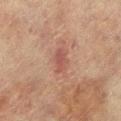Notes:
• lesion diameter — ≈2.5 mm
• patient — female, aged 78–82
• illumination — cross-polarized
• imaging modality — 15 mm crop, total-body photography
• location — the left lower leg